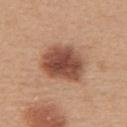Impression: This lesion was catalogued during total-body skin photography and was not selected for biopsy. Context: On the upper back. A close-up tile cropped from a whole-body skin photograph, about 15 mm across. A female subject in their mid-40s.